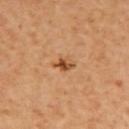Part of a total-body skin-imaging series; this lesion was reviewed on a skin check and was not flagged for biopsy. A roughly 15 mm field-of-view crop from a total-body skin photograph. A female patient. This is a cross-polarized tile. The lesion's longest dimension is about 2.5 mm. Located on the upper back.